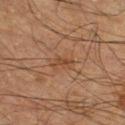A male patient, about 60 years old.
A close-up tile cropped from a whole-body skin photograph, about 15 mm across.
The lesion is on the right thigh.
Approximately 2.5 mm at its widest.